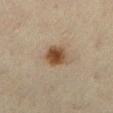- biopsy status — imaged on a skin check; not biopsied
- TBP lesion metrics — a lesion area of about 7.5 mm²; roughly 12 lightness units darker than nearby skin and a normalized border contrast of about 10.5; border irregularity of about 2 on a 0–10 scale, a within-lesion color-variation index near 4/10, and a peripheral color-asymmetry measure near 1; a classifier nevus-likeness of about 100/100 and a detector confidence of about 100 out of 100 that the crop contains a lesion
- size — about 3.5 mm
- site — the left lower leg
- image — 15 mm crop, total-body photography
- subject — female, in their mid- to late 60s